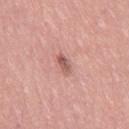Part of a total-body skin-imaging series; this lesion was reviewed on a skin check and was not flagged for biopsy.
The patient is a female aged 63 to 67.
Located on the right thigh.
This image is a 15 mm lesion crop taken from a total-body photograph.